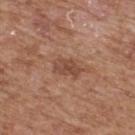On the upper back.
Cropped from a total-body skin-imaging series; the visible field is about 15 mm.
A male patient in their mid-60s.
Imaged with white-light lighting.